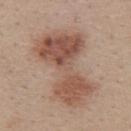Impression: Imaged during a routine full-body skin examination; the lesion was not biopsied and no histopathology is available. Image and clinical context: This image is a 15 mm lesion crop taken from a total-body photograph. The lesion-visualizer software estimated a footprint of about 38 mm², an outline eccentricity of about 0.85 (0 = round, 1 = elongated), and a shape-asymmetry score of about 0.35 (0 = symmetric). It also reported a border-irregularity index near 5/10, a within-lesion color-variation index near 6.5/10, and a peripheral color-asymmetry measure near 2.5. A female subject, aged 33 to 37. The lesion's longest dimension is about 9.5 mm. Captured under white-light illumination. The lesion is located on the upper back.